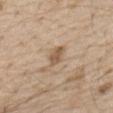The lesion was photographed on a routine skin check and not biopsied; there is no pathology result.
A 15 mm close-up extracted from a 3D total-body photography capture.
The lesion is located on the abdomen.
The lesion's longest dimension is about 3 mm.
This is a white-light tile.
Automated tile analysis of the lesion measured a mean CIELAB color near L≈56 a*≈16 b*≈31, a lesion–skin lightness drop of about 11, and a lesion-to-skin contrast of about 7.5 (normalized; higher = more distinct). It also reported a border-irregularity index near 2/10, a within-lesion color-variation index near 1.5/10, and peripheral color asymmetry of about 0.5. The software also gave a classifier nevus-likeness of about 10/100.
A male subject, in their 70s.